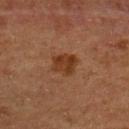follow-up=total-body-photography surveillance lesion; no biopsy
tile lighting=cross-polarized
patient=female, aged around 50
body site=the upper back
lesion diameter=~3 mm (longest diameter)
automated lesion analysis=a footprint of about 6.5 mm², a shape eccentricity near 0.45, and a symmetry-axis asymmetry near 0.25
image source=15 mm crop, total-body photography